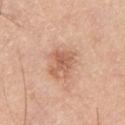Impression:
Part of a total-body skin-imaging series; this lesion was reviewed on a skin check and was not flagged for biopsy.
Image and clinical context:
Cropped from a total-body skin-imaging series; the visible field is about 15 mm. An algorithmic analysis of the crop reported about 10 CIELAB-L* units darker than the surrounding skin and a normalized border contrast of about 6.5. The software also gave a border-irregularity index near 5.5/10, a within-lesion color-variation index near 2.5/10, and a peripheral color-asymmetry measure near 1. It also reported a classifier nevus-likeness of about 30/100 and a lesion-detection confidence of about 100/100. The lesion is located on the chest. A male patient, approximately 45 years of age.